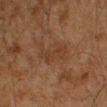Recorded during total-body skin imaging; not selected for excision or biopsy.